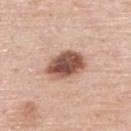| feature | finding |
|---|---|
| notes | no biopsy performed (imaged during a skin exam) |
| image-analysis metrics | a lesion area of about 13 mm², a shape eccentricity near 0.7, and a symmetry-axis asymmetry near 0.1; a classifier nevus-likeness of about 85/100 |
| tile lighting | white-light |
| patient | male, aged 58 to 62 |
| size | ≈5 mm |
| site | the upper back |
| image source | ~15 mm tile from a whole-body skin photo |